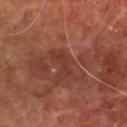Clinical impression: Part of a total-body skin-imaging series; this lesion was reviewed on a skin check and was not flagged for biopsy. Image and clinical context: Cropped from a total-body skin-imaging series; the visible field is about 15 mm. The patient is a male aged around 65. From the chest. Longest diameter approximately 3 mm.